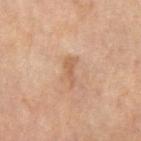Case summary:
* workup · no biopsy performed (imaged during a skin exam)
* lesion diameter · ≈3.5 mm
* image source · ~15 mm crop, total-body skin-cancer survey
* site · the right upper arm
* lighting · cross-polarized
* subject · female, approximately 60 years of age